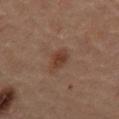follow-up: total-body-photography surveillance lesion; no biopsy | imaging modality: 15 mm crop, total-body photography | site: the mid back | tile lighting: cross-polarized | subject: male, in their 60s.